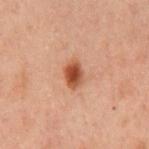biopsy_status: not biopsied; imaged during a skin examination
image:
  source: total-body photography crop
  field_of_view_mm: 15
automated_metrics:
  area_mm2_approx: 5.5
  eccentricity: 0.7
  shape_asymmetry: 0.2
  vs_skin_contrast_norm: 10.0
  border_irregularity_0_10: 1.5
  color_variation_0_10: 5.0
  peripheral_color_asymmetry: 1.5
  nevus_likeness_0_100: 100
  lesion_detection_confidence_0_100: 100
lighting: cross-polarized
site: chest
patient:
  sex: male
  age_approx: 60
lesion_size:
  long_diameter_mm_approx: 3.0